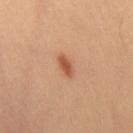location: the right thigh | acquisition: ~15 mm crop, total-body skin-cancer survey | lesion size: ~3 mm (longest diameter) | lighting: cross-polarized illumination | subject: male, aged 38–42.